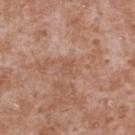| field | value |
|---|---|
| biopsy status | total-body-photography surveillance lesion; no biopsy |
| acquisition | ~15 mm tile from a whole-body skin photo |
| illumination | white-light illumination |
| body site | the upper back |
| patient | male, aged 43–47 |